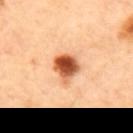biopsy status: no biopsy performed (imaged during a skin exam) | automated lesion analysis: a mean CIELAB color near L≈57 a*≈30 b*≈41, about 22 CIELAB-L* units darker than the surrounding skin, and a normalized lesion–skin contrast near 13 | subject: male, approximately 65 years of age | lighting: cross-polarized illumination | diameter: ≈3.5 mm | location: the mid back | acquisition: ~15 mm tile from a whole-body skin photo.